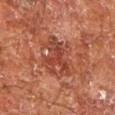Case summary:
* biopsy status — catalogued during a skin exam; not biopsied
* image — total-body-photography crop, ~15 mm field of view
* patient — male, aged 63–67
* anatomic site — the leg
* lesion size — ≈5 mm